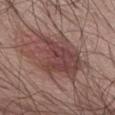workup = imaged on a skin check; not biopsied
diameter = about 7.5 mm
imaging modality = ~15 mm crop, total-body skin-cancer survey
body site = the abdomen
patient = male, aged 68–72